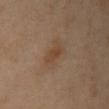{"biopsy_status": "not biopsied; imaged during a skin examination", "lesion_size": {"long_diameter_mm_approx": 3.0}, "site": "left arm", "image": {"source": "total-body photography crop", "field_of_view_mm": 15}, "automated_metrics": {"shape_asymmetry": 0.15, "cielab_L": 43, "cielab_a": 17, "cielab_b": 30, "vs_skin_darker_L": 6.0, "vs_skin_contrast_norm": 6.0}, "patient": {"sex": "female", "age_approx": 40}}